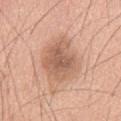Q: How was the tile lit?
A: white-light
Q: Where on the body is the lesion?
A: the mid back
Q: How was this image acquired?
A: total-body-photography crop, ~15 mm field of view
Q: What are the patient's age and sex?
A: male, aged 58 to 62